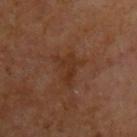Impression: No biopsy was performed on this lesion — it was imaged during a full skin examination and was not determined to be concerning. Acquisition and patient details: The lesion's longest dimension is about 4 mm. This image is a 15 mm lesion crop taken from a total-body photograph. A male subject, aged 63–67. On the arm. This is a cross-polarized tile. The lesion-visualizer software estimated a border-irregularity index near 5.5/10, internal color variation of about 2 on a 0–10 scale, and radial color variation of about 0.5.